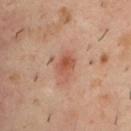Q: Was this lesion biopsied?
A: imaged on a skin check; not biopsied
Q: What did automated image analysis measure?
A: a border-irregularity index near 2/10 and radial color variation of about 1.5; a classifier nevus-likeness of about 95/100 and a detector confidence of about 100 out of 100 that the crop contains a lesion
Q: What kind of image is this?
A: ~15 mm crop, total-body skin-cancer survey
Q: Lesion size?
A: ≈3 mm
Q: What are the patient's age and sex?
A: male, aged around 40
Q: Lesion location?
A: the upper back